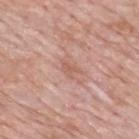biopsy_status: not biopsied; imaged during a skin examination
patient:
  sex: male
  age_approx: 60
lighting: white-light
automated_metrics:
  vs_skin_darker_L: 7.0
  vs_skin_contrast_norm: 5.5
  border_irregularity_0_10: 5.0
  color_variation_0_10: 0.0
  peripheral_color_asymmetry: 0.0
site: mid back
image:
  source: total-body photography crop
  field_of_view_mm: 15